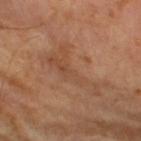Assessment: Recorded during total-body skin imaging; not selected for excision or biopsy. Acquisition and patient details: Measured at roughly 7.5 mm in maximum diameter. A male subject, aged 63–67. A 15 mm crop from a total-body photograph taken for skin-cancer surveillance. Automated tile analysis of the lesion measured a border-irregularity index near 9.5/10, a color-variation rating of about 3/10, and radial color variation of about 1. Captured under cross-polarized illumination.